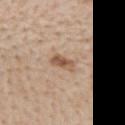The lesion was tiled from a total-body skin photograph and was not biopsied.
A region of skin cropped from a whole-body photographic capture, roughly 15 mm wide.
Captured under white-light illumination.
Measured at roughly 3 mm in maximum diameter.
A male patient, approximately 60 years of age.
Located on the mid back.